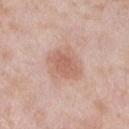The lesion was photographed on a routine skin check and not biopsied; there is no pathology result. A close-up tile cropped from a whole-body skin photograph, about 15 mm across. Located on the left lower leg. A female subject aged 58–62.